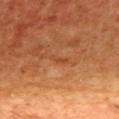Recorded during total-body skin imaging; not selected for excision or biopsy. This image is a 15 mm lesion crop taken from a total-body photograph. The patient is a female in their 40s. The lesion is on the upper back.